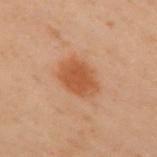automated lesion analysis = internal color variation of about 2.5 on a 0–10 scale
diameter = ≈4.5 mm
subject = female, in their 60s
body site = the upper back
lighting = cross-polarized
image = ~15 mm tile from a whole-body skin photo